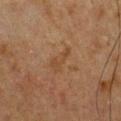patient: male, about 50 years old | location: the front of the torso | TBP lesion metrics: an eccentricity of roughly 0.9 and a shape-asymmetry score of about 0.5 (0 = symmetric) | image: ~15 mm tile from a whole-body skin photo | lesion diameter: ~3.5 mm (longest diameter).